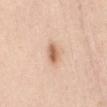Notes:
* follow-up — imaged on a skin check; not biopsied
* size — about 3 mm
* subject — female, aged around 40
* anatomic site — the abdomen
* illumination — white-light illumination
* imaging modality — total-body-photography crop, ~15 mm field of view